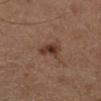biopsy_status: not biopsied; imaged during a skin examination
site: left lower leg
image:
  source: total-body photography crop
  field_of_view_mm: 15
patient:
  sex: male
  age_approx: 60